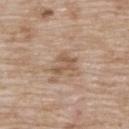Q: Is there a histopathology result?
A: no biopsy performed (imaged during a skin exam)
Q: Who is the patient?
A: female, in their mid- to late 70s
Q: What is the imaging modality?
A: 15 mm crop, total-body photography
Q: Illumination type?
A: white-light illumination
Q: What is the anatomic site?
A: the upper back